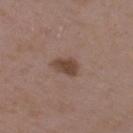This lesion was catalogued during total-body skin photography and was not selected for biopsy. The recorded lesion diameter is about 3 mm. Automated image analysis of the tile measured a lesion color around L≈43 a*≈17 b*≈25 in CIELAB, roughly 11 lightness units darker than nearby skin, and a normalized lesion–skin contrast near 8.5. And it measured an automated nevus-likeness rating near 80 out of 100 and a lesion-detection confidence of about 100/100. Located on the right upper arm. This is a white-light tile. Cropped from a whole-body photographic skin survey; the tile spans about 15 mm. The patient is a female in their mid-30s.